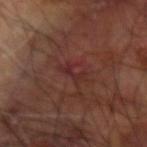Assessment: The lesion was photographed on a routine skin check and not biopsied; there is no pathology result. Acquisition and patient details: A male subject about 60 years old. The tile uses cross-polarized illumination. On the arm. Cropped from a total-body skin-imaging series; the visible field is about 15 mm. Approximately 3.5 mm at its widest.